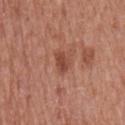follow-up: no biopsy performed (imaged during a skin exam); patient: male, in their mid-60s; image source: 15 mm crop, total-body photography; location: the chest.